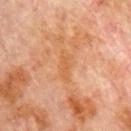This image is a 15 mm lesion crop taken from a total-body photograph.
From the chest.
The recorded lesion diameter is about 3.5 mm.
A male subject, roughly 80 years of age.